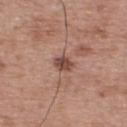No biopsy was performed on this lesion — it was imaged during a full skin examination and was not determined to be concerning. The patient is a male approximately 65 years of age. A roughly 15 mm field-of-view crop from a total-body skin photograph. The lesion is located on the upper back.